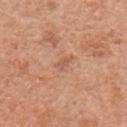Captured during whole-body skin photography for melanoma surveillance; the lesion was not biopsied.
The lesion is on the chest.
Cropped from a total-body skin-imaging series; the visible field is about 15 mm.
A male subject aged 63 to 67.